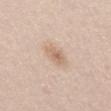Case summary:
• follow-up — catalogued during a skin exam; not biopsied
• automated lesion analysis — a border-irregularity index near 2.5/10, a within-lesion color-variation index near 3/10, and radial color variation of about 1; lesion-presence confidence of about 100/100
• site — the abdomen
• lighting — white-light
• patient — male, aged 28–32
• diameter — ~3 mm (longest diameter)
• imaging modality — 15 mm crop, total-body photography